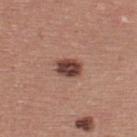Case summary:
* biopsy status: total-body-photography surveillance lesion; no biopsy
* lighting: white-light illumination
* acquisition: total-body-photography crop, ~15 mm field of view
* automated metrics: a footprint of about 6 mm² and a shape eccentricity near 0.65; a border-irregularity rating of about 1/10, a color-variation rating of about 4/10, and radial color variation of about 1.5; a classifier nevus-likeness of about 75/100 and a detector confidence of about 100 out of 100 that the crop contains a lesion
* location: the upper back
* size: ~3 mm (longest diameter)
* patient: male, aged 38 to 42